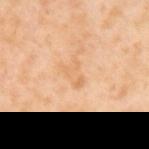Notes:
* workup: catalogued during a skin exam; not biopsied
* body site: the mid back
* patient: female, about 55 years old
* image source: 15 mm crop, total-body photography
* automated lesion analysis: an average lesion color of about L≈71 a*≈21 b*≈41 (CIELAB), a lesion–skin lightness drop of about 6, and a normalized lesion–skin contrast near 4.5
* diameter: ~3 mm (longest diameter)
* tile lighting: cross-polarized